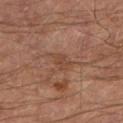{
  "biopsy_status": "not biopsied; imaged during a skin examination",
  "image": {
    "source": "total-body photography crop",
    "field_of_view_mm": 15
  },
  "patient": {
    "sex": "male",
    "age_approx": 65
  },
  "site": "left lower leg",
  "automated_metrics": {
    "area_mm2_approx": 3.5,
    "eccentricity": 0.75,
    "shape_asymmetry": 0.3,
    "cielab_L": 43,
    "cielab_a": 20,
    "cielab_b": 29,
    "vs_skin_darker_L": 6.0,
    "vs_skin_contrast_norm": 5.5
  },
  "lighting": "cross-polarized"
}